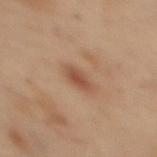The lesion was photographed on a routine skin check and not biopsied; there is no pathology result. A lesion tile, about 15 mm wide, cut from a 3D total-body photograph. A female subject, aged 53 to 57. On the upper back. This is a cross-polarized tile.